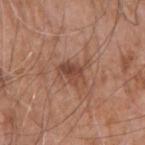<lesion>
<automated_metrics>
  <area_mm2_approx>6.0</area_mm2_approx>
  <eccentricity>0.7</eccentricity>
  <shape_asymmetry>0.4</shape_asymmetry>
  <vs_skin_darker_L>9.0</vs_skin_darker_L>
  <vs_skin_contrast_norm>7.0</vs_skin_contrast_norm>
  <border_irregularity_0_10>5.0</border_irregularity_0_10>
  <color_variation_0_10>3.5</color_variation_0_10>
  <peripheral_color_asymmetry>1.0</peripheral_color_asymmetry>
  <nevus_likeness_0_100>65</nevus_likeness_0_100>
  <lesion_detection_confidence_0_100>100</lesion_detection_confidence_0_100>
</automated_metrics>
<lighting>white-light</lighting>
<patient>
  <sex>male</sex>
  <age_approx>75</age_approx>
</patient>
<lesion_size>
  <long_diameter_mm_approx>3.5</long_diameter_mm_approx>
</lesion_size>
<site>right upper arm</site>
<image>
  <source>total-body photography crop</source>
  <field_of_view_mm>15</field_of_view_mm>
</image>
</lesion>